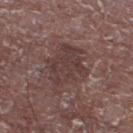Q: Was a biopsy performed?
A: total-body-photography surveillance lesion; no biopsy
Q: What is the anatomic site?
A: the right lower leg
Q: What is the imaging modality?
A: ~15 mm tile from a whole-body skin photo
Q: What are the patient's age and sex?
A: male, aged around 65
Q: What lighting was used for the tile?
A: white-light illumination